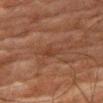Impression: This lesion was catalogued during total-body skin photography and was not selected for biopsy. Clinical summary: The tile uses cross-polarized illumination. From the right thigh. Automated tile analysis of the lesion measured a lesion area of about 3.5 mm², an eccentricity of roughly 0.8, and a symmetry-axis asymmetry near 0.3. It also reported an average lesion color of about L≈31 a*≈18 b*≈25 (CIELAB) and a lesion-to-skin contrast of about 5 (normalized; higher = more distinct). Approximately 2.5 mm at its widest. A male patient, roughly 80 years of age. A region of skin cropped from a whole-body photographic capture, roughly 15 mm wide.